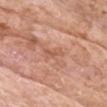follow-up: no biopsy performed (imaged during a skin exam)
patient: male, aged around 75
image: ~15 mm tile from a whole-body skin photo
illumination: white-light illumination
size: ≈2.5 mm
location: the head or neck